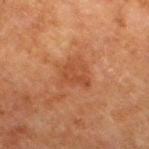Clinical impression:
Part of a total-body skin-imaging series; this lesion was reviewed on a skin check and was not flagged for biopsy.
Background:
The recorded lesion diameter is about 3.5 mm. The lesion is on the right thigh. The tile uses cross-polarized illumination. A close-up tile cropped from a whole-body skin photograph, about 15 mm across. A male patient, aged approximately 80. The lesion-visualizer software estimated a footprint of about 8 mm², an eccentricity of roughly 0.45, and a symmetry-axis asymmetry near 0.35. The software also gave a mean CIELAB color near L≈38 a*≈22 b*≈30, roughly 6 lightness units darker than nearby skin, and a normalized lesion–skin contrast near 5.5. The analysis additionally found border irregularity of about 3.5 on a 0–10 scale and a within-lesion color-variation index near 2/10.